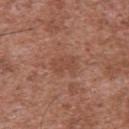{"patient": {"sex": "male", "age_approx": 45}, "lighting": "white-light", "image": {"source": "total-body photography crop", "field_of_view_mm": 15}, "automated_metrics": {"area_mm2_approx": 5.0, "eccentricity": 0.75, "shape_asymmetry": 0.2, "border_irregularity_0_10": 2.5, "color_variation_0_10": 1.5, "peripheral_color_asymmetry": 0.5}, "site": "upper back", "lesion_size": {"long_diameter_mm_approx": 3.0}}